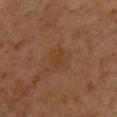The lesion was tiled from a total-body skin photograph and was not biopsied. The total-body-photography lesion software estimated a lesion color around L≈30 a*≈16 b*≈27 in CIELAB, roughly 4 lightness units darker than nearby skin, and a normalized border contrast of about 5. The analysis additionally found a classifier nevus-likeness of about 0/100 and lesion-presence confidence of about 100/100. Longest diameter approximately 2.5 mm. The tile uses cross-polarized illumination. A lesion tile, about 15 mm wide, cut from a 3D total-body photograph. The lesion is on the chest. The subject is a male about 65 years old.